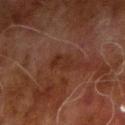On the arm.
This image is a 15 mm lesion crop taken from a total-body photograph.
A male subject, aged 68–72.